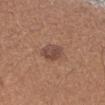Part of a total-body skin-imaging series; this lesion was reviewed on a skin check and was not flagged for biopsy.
A lesion tile, about 15 mm wide, cut from a 3D total-body photograph.
Located on the left forearm.
The recorded lesion diameter is about 3.5 mm.
Automated tile analysis of the lesion measured a symmetry-axis asymmetry near 0.15. The analysis additionally found a lesion color around L≈46 a*≈19 b*≈25 in CIELAB and a lesion–skin lightness drop of about 10.
A female patient aged 43 to 47.
The tile uses white-light illumination.